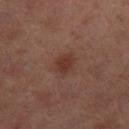follow-up=catalogued during a skin exam; not biopsied
image=15 mm crop, total-body photography
location=the right thigh
illumination=cross-polarized
diameter=~3 mm (longest diameter)
patient=female, aged 58 to 62